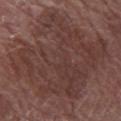This lesion was catalogued during total-body skin photography and was not selected for biopsy. The recorded lesion diameter is about 11 mm. A female subject approximately 70 years of age. From the arm. A 15 mm close-up extracted from a 3D total-body photography capture. This is a white-light tile.